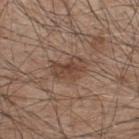Assessment:
Captured during whole-body skin photography for melanoma surveillance; the lesion was not biopsied.
Image and clinical context:
The lesion is on the upper back. A 15 mm crop from a total-body photograph taken for skin-cancer surveillance. Measured at roughly 4 mm in maximum diameter. A male subject, about 45 years old. Automated tile analysis of the lesion measured an average lesion color of about L≈44 a*≈18 b*≈26 (CIELAB) and about 9 CIELAB-L* units darker than the surrounding skin. And it measured border irregularity of about 3 on a 0–10 scale, a color-variation rating of about 3/10, and peripheral color asymmetry of about 1.